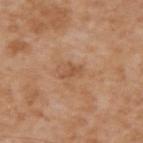• workup · total-body-photography surveillance lesion; no biopsy
• patient · male, aged 63–67
• image source · ~15 mm tile from a whole-body skin photo
• site · the upper back
• image-analysis metrics · an area of roughly 3 mm² and a shape eccentricity near 0.8; a border-irregularity rating of about 5/10, a color-variation rating of about 0.5/10, and a peripheral color-asymmetry measure near 0; a nevus-likeness score of about 0/100 and a detector confidence of about 100 out of 100 that the crop contains a lesion
• illumination · white-light
• lesion size · about 2.5 mm